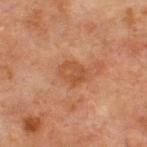follow-up: no biopsy performed (imaged during a skin exam)
size: about 3 mm
image: 15 mm crop, total-body photography
image-analysis metrics: an area of roughly 6 mm², an outline eccentricity of about 0.65 (0 = round, 1 = elongated), and a symmetry-axis asymmetry near 0.15; a nevus-likeness score of about 0/100 and lesion-presence confidence of about 100/100
patient: male, aged approximately 65
location: the front of the torso
illumination: cross-polarized